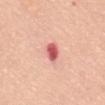<case>
<biopsy_status>not biopsied; imaged during a skin examination</biopsy_status>
</case>